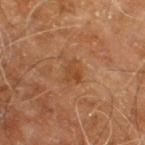Clinical impression:
No biopsy was performed on this lesion — it was imaged during a full skin examination and was not determined to be concerning.
Acquisition and patient details:
Approximately 2.5 mm at its widest. Located on the right leg. A close-up tile cropped from a whole-body skin photograph, about 15 mm across. A male patient aged 58–62. The tile uses cross-polarized illumination. An algorithmic analysis of the crop reported border irregularity of about 4 on a 0–10 scale and a peripheral color-asymmetry measure near 0.5.